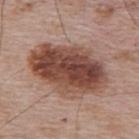The lesion was photographed on a routine skin check and not biopsied; there is no pathology result. Captured under white-light illumination. Measured at roughly 9.5 mm in maximum diameter. A male subject roughly 65 years of age. The total-body-photography lesion software estimated a mean CIELAB color near L≈46 a*≈21 b*≈26, about 16 CIELAB-L* units darker than the surrounding skin, and a normalized lesion–skin contrast near 11. And it measured a border-irregularity index near 2/10, internal color variation of about 8.5 on a 0–10 scale, and a peripheral color-asymmetry measure near 3. And it measured a nevus-likeness score of about 95/100 and a lesion-detection confidence of about 100/100. Cropped from a whole-body photographic skin survey; the tile spans about 15 mm. On the upper back.